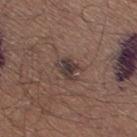The lesion was tiled from a total-body skin photograph and was not biopsied. The lesion's longest dimension is about 3 mm. A male patient aged 33 to 37. Automated image analysis of the tile measured an outline eccentricity of about 0.75 (0 = round, 1 = elongated) and two-axis asymmetry of about 0.3. The analysis additionally found roughly 10 lightness units darker than nearby skin and a normalized lesion–skin contrast near 9.5. The tile uses white-light illumination. A close-up tile cropped from a whole-body skin photograph, about 15 mm across. The lesion is located on the right thigh.